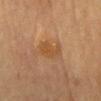follow-up: imaged on a skin check; not biopsied | patient: female, roughly 60 years of age | image source: ~15 mm tile from a whole-body skin photo | lighting: cross-polarized | location: the chest | lesion diameter: about 3 mm.